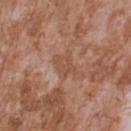| key | value |
|---|---|
| biopsy status | no biopsy performed (imaged during a skin exam) |
| location | the upper back |
| subject | male, in their mid-40s |
| size | about 3 mm |
| imaging modality | total-body-photography crop, ~15 mm field of view |
| tile lighting | white-light |